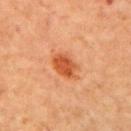Assessment: No biopsy was performed on this lesion — it was imaged during a full skin examination and was not determined to be concerning. Acquisition and patient details: The subject is a female aged 63 to 67. Cropped from a total-body skin-imaging series; the visible field is about 15 mm. The lesion is located on the left upper arm.